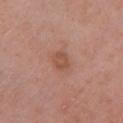Captured during whole-body skin photography for melanoma surveillance; the lesion was not biopsied.
The lesion is located on the left lower leg.
An algorithmic analysis of the crop reported a mean CIELAB color near L≈52 a*≈23 b*≈29, a lesion–skin lightness drop of about 7, and a normalized border contrast of about 6. It also reported a border-irregularity index near 1/10, a within-lesion color-variation index near 1.5/10, and a peripheral color-asymmetry measure near 0.5. It also reported an automated nevus-likeness rating near 55 out of 100 and a lesion-detection confidence of about 100/100.
This is a white-light tile.
Approximately 2.5 mm at its widest.
A male patient approximately 80 years of age.
Cropped from a total-body skin-imaging series; the visible field is about 15 mm.